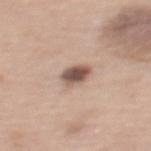Findings:
• workup · total-body-photography surveillance lesion; no biopsy
• lighting · white-light
• subject · female, approximately 60 years of age
• location · the upper back
• TBP lesion metrics · a lesion area of about 5 mm², an outline eccentricity of about 0.55 (0 = round, 1 = elongated), and a shape-asymmetry score of about 0.15 (0 = symmetric); an average lesion color of about L≈52 a*≈18 b*≈23 (CIELAB) and about 17 CIELAB-L* units darker than the surrounding skin; a nevus-likeness score of about 70/100 and a lesion-detection confidence of about 100/100
• size · about 2.5 mm
• imaging modality · 15 mm crop, total-body photography